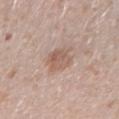The lesion is on the right forearm. A female patient about 30 years old. A region of skin cropped from a whole-body photographic capture, roughly 15 mm wide.